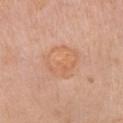Findings:
– follow-up · imaged on a skin check; not biopsied
– lighting · white-light
– patient · female, about 70 years old
– site · the chest
– imaging modality · 15 mm crop, total-body photography